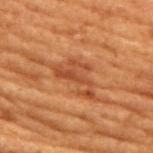The lesion was tiled from a total-body skin photograph and was not biopsied. Located on the front of the torso. This image is a 15 mm lesion crop taken from a total-body photograph. A female subject, in their 80s.